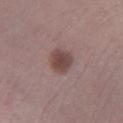Findings:
– patient — male, aged 58–62
– lighting — white-light illumination
– location — the left lower leg
– lesion diameter — ~3 mm (longest diameter)
– imaging modality — total-body-photography crop, ~15 mm field of view